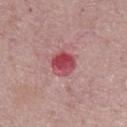Q: Was this lesion biopsied?
A: imaged on a skin check; not biopsied
Q: What is the imaging modality?
A: total-body-photography crop, ~15 mm field of view
Q: Lesion location?
A: the chest
Q: Who is the patient?
A: male, in their 70s